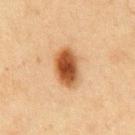{
  "biopsy_status": "not biopsied; imaged during a skin examination",
  "image": {
    "source": "total-body photography crop",
    "field_of_view_mm": 15
  },
  "patient": {
    "sex": "male",
    "age_approx": 75
  },
  "lesion_size": {
    "long_diameter_mm_approx": 4.5
  },
  "automated_metrics": {
    "cielab_L": 44,
    "cielab_a": 20,
    "cielab_b": 33,
    "border_irregularity_0_10": 1.5,
    "color_variation_0_10": 6.5,
    "peripheral_color_asymmetry": 2.0,
    "nevus_likeness_0_100": 100
  },
  "lighting": "cross-polarized",
  "site": "front of the torso"
}